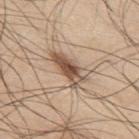The lesion is on the upper back. A region of skin cropped from a whole-body photographic capture, roughly 15 mm wide. A male subject, aged around 45.